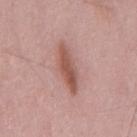This lesion was catalogued during total-body skin photography and was not selected for biopsy.
The recorded lesion diameter is about 5.5 mm.
Imaged with white-light lighting.
On the mid back.
The subject is a male roughly 50 years of age.
A close-up tile cropped from a whole-body skin photograph, about 15 mm across.